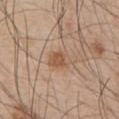Q: Was this lesion biopsied?
A: total-body-photography surveillance lesion; no biopsy
Q: What are the patient's age and sex?
A: male, about 55 years old
Q: What kind of image is this?
A: total-body-photography crop, ~15 mm field of view
Q: What is the lesion's diameter?
A: ~3.5 mm (longest diameter)
Q: Lesion location?
A: the upper back
Q: Automated lesion metrics?
A: a lesion color around L≈55 a*≈19 b*≈32 in CIELAB, a lesion–skin lightness drop of about 9, and a normalized lesion–skin contrast near 6.5; a nevus-likeness score of about 70/100 and a lesion-detection confidence of about 100/100